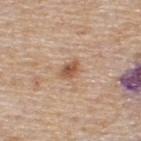Q: Was this lesion biopsied?
A: no biopsy performed (imaged during a skin exam)
Q: Where on the body is the lesion?
A: the upper back
Q: What lighting was used for the tile?
A: white-light illumination
Q: What is the lesion's diameter?
A: ≈2.5 mm
Q: Automated lesion metrics?
A: a border-irregularity rating of about 2.5/10, a color-variation rating of about 4.5/10, and radial color variation of about 1.5; a classifier nevus-likeness of about 90/100 and a lesion-detection confidence of about 100/100
Q: What are the patient's age and sex?
A: male, aged around 55
Q: What is the imaging modality?
A: ~15 mm tile from a whole-body skin photo